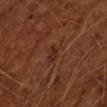A region of skin cropped from a whole-body photographic capture, roughly 15 mm wide. A male patient approximately 65 years of age.